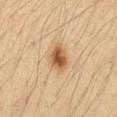| field | value |
|---|---|
| tile lighting | cross-polarized |
| site | the abdomen |
| TBP lesion metrics | an eccentricity of roughly 0.7; internal color variation of about 4 on a 0–10 scale and peripheral color asymmetry of about 1.5 |
| lesion diameter | about 3.5 mm |
| acquisition | ~15 mm tile from a whole-body skin photo |
| patient | male, aged approximately 35 |